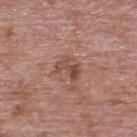Background:
A close-up tile cropped from a whole-body skin photograph, about 15 mm across. Located on the upper back. A male patient aged around 75. An algorithmic analysis of the crop reported an average lesion color of about L≈48 a*≈22 b*≈26 (CIELAB), about 9 CIELAB-L* units darker than the surrounding skin, and a lesion-to-skin contrast of about 7 (normalized; higher = more distinct). And it measured border irregularity of about 6.5 on a 0–10 scale, a color-variation rating of about 1.5/10, and peripheral color asymmetry of about 0.5. And it measured a classifier nevus-likeness of about 0/100 and a lesion-detection confidence of about 100/100. The tile uses white-light illumination.